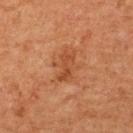<record>
  <biopsy_status>not biopsied; imaged during a skin examination</biopsy_status>
  <lesion_size>
    <long_diameter_mm_approx>3.5</long_diameter_mm_approx>
  </lesion_size>
  <site>upper back</site>
  <automated_metrics>
    <area_mm2_approx>4.5</area_mm2_approx>
    <cielab_L>40</cielab_L>
    <cielab_a>24</cielab_a>
    <cielab_b>34</cielab_b>
    <vs_skin_darker_L>7.0</vs_skin_darker_L>
    <vs_skin_contrast_norm>6.5</vs_skin_contrast_norm>
    <border_irregularity_0_10>4.5</border_irregularity_0_10>
    <color_variation_0_10>1.0</color_variation_0_10>
    <peripheral_color_asymmetry>0.5</peripheral_color_asymmetry>
  </automated_metrics>
  <lighting>cross-polarized</lighting>
  <patient>
    <sex>female</sex>
    <age_approx>50</age_approx>
  </patient>
  <image>
    <source>total-body photography crop</source>
    <field_of_view_mm>15</field_of_view_mm>
  </image>
</record>